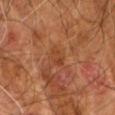Clinical impression:
Captured during whole-body skin photography for melanoma surveillance; the lesion was not biopsied.
Clinical summary:
The lesion's longest dimension is about 2.5 mm. The tile uses cross-polarized illumination. A lesion tile, about 15 mm wide, cut from a 3D total-body photograph. A male subject, aged approximately 60. The lesion is located on the arm.